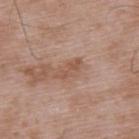The lesion was tiled from a total-body skin photograph and was not biopsied.
An algorithmic analysis of the crop reported a border-irregularity rating of about 6/10 and internal color variation of about 0 on a 0–10 scale.
The lesion is on the upper back.
A 15 mm close-up extracted from a 3D total-body photography capture.
A male patient, aged 48 to 52.
Measured at roughly 3 mm in maximum diameter.